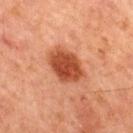biopsy status — no biopsy performed (imaged during a skin exam) | lesion size — about 4.5 mm | image source — total-body-photography crop, ~15 mm field of view | patient — male, in their mid- to late 60s | location — the front of the torso | illumination — cross-polarized.